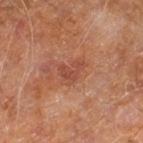Q: Was this lesion biopsied?
A: no biopsy performed (imaged during a skin exam)
Q: Who is the patient?
A: male, about 60 years old
Q: Lesion location?
A: the right leg
Q: How was this image acquired?
A: total-body-photography crop, ~15 mm field of view
Q: Automated lesion metrics?
A: an outline eccentricity of about 0.7 (0 = round, 1 = elongated); a lesion color around L≈45 a*≈26 b*≈30 in CIELAB, a lesion–skin lightness drop of about 7, and a normalized lesion–skin contrast near 5.5
Q: How was the tile lit?
A: cross-polarized